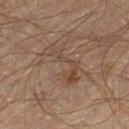This lesion was catalogued during total-body skin photography and was not selected for biopsy.
A 15 mm crop from a total-body photograph taken for skin-cancer surveillance.
Located on the left thigh.
The patient is a male aged 58–62.